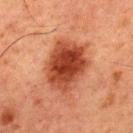Imaged during a routine full-body skin examination; the lesion was not biopsied and no histopathology is available. Cropped from a whole-body photographic skin survey; the tile spans about 15 mm. A male subject, aged 58 to 62. The lesion is located on the back. The lesion's longest dimension is about 6 mm. Captured under cross-polarized illumination.